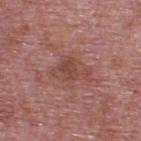Imaged during a routine full-body skin examination; the lesion was not biopsied and no histopathology is available. The subject is a male aged around 75. Located on the upper back. A 15 mm crop from a total-body photograph taken for skin-cancer surveillance. This is a white-light tile. Automated tile analysis of the lesion measured a footprint of about 8 mm² and an eccentricity of roughly 0.8. The software also gave a mean CIELAB color near L≈46 a*≈23 b*≈25, about 7 CIELAB-L* units darker than the surrounding skin, and a normalized border contrast of about 6. About 4.5 mm across.